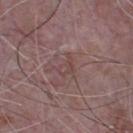Q: What is the anatomic site?
A: the chest
Q: What lighting was used for the tile?
A: white-light illumination
Q: Lesion size?
A: about 3 mm
Q: What is the imaging modality?
A: 15 mm crop, total-body photography
Q: Who is the patient?
A: male, approximately 65 years of age